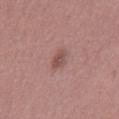Impression: The lesion was photographed on a routine skin check and not biopsied; there is no pathology result. Background: Captured under white-light illumination. Approximately 3.5 mm at its widest. From the left thigh. The total-body-photography lesion software estimated a footprint of about 4.5 mm² and a shape-asymmetry score of about 0.25 (0 = symmetric). The analysis additionally found a mean CIELAB color near L≈51 a*≈21 b*≈22 and a normalized border contrast of about 6. It also reported an automated nevus-likeness rating near 5 out of 100 and a detector confidence of about 100 out of 100 that the crop contains a lesion. The subject is a female about 50 years old. A 15 mm close-up tile from a total-body photography series done for melanoma screening.